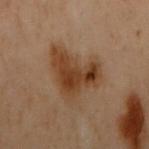The lesion was photographed on a routine skin check and not biopsied; there is no pathology result.
A female patient, aged 58–62.
A close-up tile cropped from a whole-body skin photograph, about 15 mm across.
The lesion is on the upper back.
About 6.5 mm across.